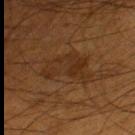workup: catalogued during a skin exam; not biopsied | subject: male, about 60 years old | acquisition: ~15 mm crop, total-body skin-cancer survey | site: the left thigh.